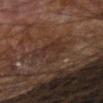workup: no biopsy performed (imaged during a skin exam)
tile lighting: cross-polarized
lesion diameter: ≈5.5 mm
image: ~15 mm tile from a whole-body skin photo
subject: male, aged around 65
body site: the left arm